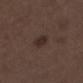workup = imaged on a skin check; not biopsied
size = ~2.5 mm (longest diameter)
tile lighting = white-light illumination
acquisition = ~15 mm tile from a whole-body skin photo
subject = male, about 50 years old
body site = the left lower leg